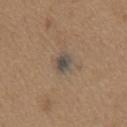– notes · total-body-photography surveillance lesion; no biopsy
– lighting · white-light illumination
– subject · male, in their mid- to late 60s
– image · ~15 mm tile from a whole-body skin photo
– lesion size · about 2.5 mm
– site · the front of the torso
– automated metrics · an area of roughly 4.5 mm², an outline eccentricity of about 0.65 (0 = round, 1 = elongated), and a shape-asymmetry score of about 0.2 (0 = symmetric); a lesion color around L≈47 a*≈8 b*≈20 in CIELAB and a lesion–skin lightness drop of about 10; an automated nevus-likeness rating near 100 out of 100 and a lesion-detection confidence of about 95/100